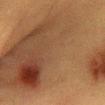The subject is a female aged 38–42. A lesion tile, about 15 mm wide, cut from a 3D total-body photograph. Automated image analysis of the tile measured a footprint of about 180 mm². The analysis additionally found a nevus-likeness score of about 40/100 and a detector confidence of about 95 out of 100 that the crop contains a lesion. The recorded lesion diameter is about 20.5 mm. This is a cross-polarized tile. Located on the abdomen.